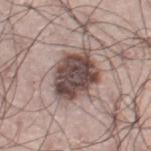follow-up = total-body-photography surveillance lesion; no biopsy
diameter = ~6 mm (longest diameter)
acquisition = total-body-photography crop, ~15 mm field of view
tile lighting = white-light
location = the leg
patient = male, approximately 70 years of age